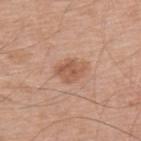A male patient, aged around 55.
Longest diameter approximately 3.5 mm.
Imaged with white-light lighting.
Located on the back.
A roughly 15 mm field-of-view crop from a total-body skin photograph.